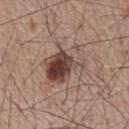The lesion was photographed on a routine skin check and not biopsied; there is no pathology result.
The lesion's longest dimension is about 5.5 mm.
The tile uses white-light illumination.
A roughly 15 mm field-of-view crop from a total-body skin photograph.
The patient is a male in their mid-60s.
The lesion is located on the front of the torso.